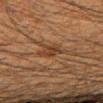Assessment: Recorded during total-body skin imaging; not selected for excision or biopsy. Clinical summary: Located on the right upper arm. A 15 mm crop from a total-body photograph taken for skin-cancer surveillance. A male patient, aged around 35.